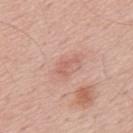Case summary:
• follow-up: total-body-photography surveillance lesion; no biopsy
• diameter: ≈2.5 mm
• imaging modality: 15 mm crop, total-body photography
• subject: male, aged around 55
• body site: the back
• TBP lesion metrics: a classifier nevus-likeness of about 0/100 and lesion-presence confidence of about 100/100